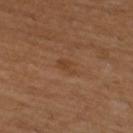Captured during whole-body skin photography for melanoma surveillance; the lesion was not biopsied.
A female subject, aged approximately 55.
This is a cross-polarized tile.
A region of skin cropped from a whole-body photographic capture, roughly 15 mm wide.
The lesion is on the left lower leg.